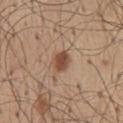{"biopsy_status": "not biopsied; imaged during a skin examination", "lighting": "white-light", "patient": {"sex": "male", "age_approx": 55}, "automated_metrics": {"area_mm2_approx": 4.5, "eccentricity": 0.65, "shape_asymmetry": 0.15, "cielab_L": 48, "cielab_a": 20, "cielab_b": 30, "vs_skin_darker_L": 13.0}, "site": "mid back", "lesion_size": {"long_diameter_mm_approx": 3.0}, "image": {"source": "total-body photography crop", "field_of_view_mm": 15}}